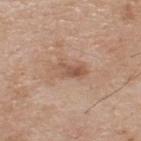The subject is a male in their mid-70s.
The total-body-photography lesion software estimated an area of roughly 5 mm², an outline eccentricity of about 0.9 (0 = round, 1 = elongated), and a shape-asymmetry score of about 0.3 (0 = symmetric). It also reported a color-variation rating of about 3.5/10 and peripheral color asymmetry of about 1.
The lesion is located on the back.
Imaged with white-light lighting.
A close-up tile cropped from a whole-body skin photograph, about 15 mm across.